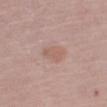<record>
  <biopsy_status>not biopsied; imaged during a skin examination</biopsy_status>
  <patient>
    <sex>male</sex>
    <age_approx>65</age_approx>
  </patient>
  <site>left upper arm</site>
  <image>
    <source>total-body photography crop</source>
    <field_of_view_mm>15</field_of_view_mm>
  </image>
</record>